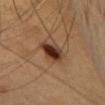Impression: Captured during whole-body skin photography for melanoma surveillance; the lesion was not biopsied. Background: Cropped from a total-body skin-imaging series; the visible field is about 15 mm. On the head or neck. Imaged with cross-polarized lighting. The lesion's longest dimension is about 3.5 mm. A female patient, in their mid- to late 30s.